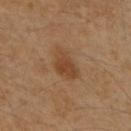Assessment:
The lesion was tiled from a total-body skin photograph and was not biopsied.
Clinical summary:
A region of skin cropped from a whole-body photographic capture, roughly 15 mm wide. From the upper back. The tile uses cross-polarized illumination. The recorded lesion diameter is about 4 mm. A male patient, in their 60s.